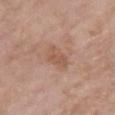<lesion>
<biopsy_status>not biopsied; imaged during a skin examination</biopsy_status>
<patient>
  <sex>female</sex>
  <age_approx>75</age_approx>
</patient>
<site>chest</site>
<image>
  <source>total-body photography crop</source>
  <field_of_view_mm>15</field_of_view_mm>
</image>
</lesion>